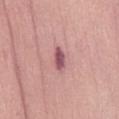The lesion was tiled from a total-body skin photograph and was not biopsied.
This is a white-light tile.
Cropped from a whole-body photographic skin survey; the tile spans about 15 mm.
From the abdomen.
A female subject aged 58–62.
About 3 mm across.